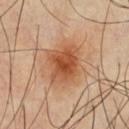Clinical summary:
Captured under cross-polarized illumination. The total-body-photography lesion software estimated a lesion area of about 11 mm², an eccentricity of roughly 0.6, and a symmetry-axis asymmetry near 0.25. The analysis additionally found roughly 12 lightness units darker than nearby skin and a lesion-to-skin contrast of about 9 (normalized; higher = more distinct). It also reported border irregularity of about 2.5 on a 0–10 scale, a color-variation rating of about 4/10, and peripheral color asymmetry of about 1. The analysis additionally found lesion-presence confidence of about 100/100. A 15 mm close-up extracted from a 3D total-body photography capture. From the chest. A male subject aged approximately 50.